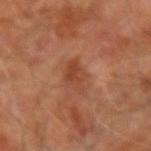<lesion>
  <biopsy_status>not biopsied; imaged during a skin examination</biopsy_status>
  <automated_metrics>
    <lesion_detection_confidence_0_100>100</lesion_detection_confidence_0_100>
  </automated_metrics>
  <patient>
    <sex>male</sex>
    <age_approx>65</age_approx>
  </patient>
  <lesion_size>
    <long_diameter_mm_approx>4.0</long_diameter_mm_approx>
  </lesion_size>
  <lighting>cross-polarized</lighting>
  <image>
    <source>total-body photography crop</source>
    <field_of_view_mm>15</field_of_view_mm>
  </image>
  <site>right forearm</site>
</lesion>